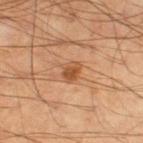This lesion was catalogued during total-body skin photography and was not selected for biopsy.
Located on the left thigh.
A roughly 15 mm field-of-view crop from a total-body skin photograph.
The patient is a male roughly 45 years of age.